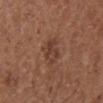Clinical impression: No biopsy was performed on this lesion — it was imaged during a full skin examination and was not determined to be concerning. Image and clinical context: The subject is a male aged 73 to 77. From the right lower leg. A 15 mm close-up tile from a total-body photography series done for melanoma screening.